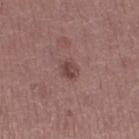Recorded during total-body skin imaging; not selected for excision or biopsy.
The patient is a male aged 43–47.
Imaged with white-light lighting.
The lesion is on the right thigh.
The lesion's longest dimension is about 2.5 mm.
Automated image analysis of the tile measured a shape-asymmetry score of about 0.25 (0 = symmetric). And it measured a classifier nevus-likeness of about 15/100 and a lesion-detection confidence of about 100/100.
A lesion tile, about 15 mm wide, cut from a 3D total-body photograph.